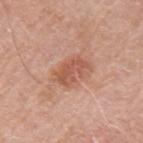Captured during whole-body skin photography for melanoma surveillance; the lesion was not biopsied. The lesion is located on the left upper arm. A lesion tile, about 15 mm wide, cut from a 3D total-body photograph. This is a white-light tile. A male patient, aged 78 to 82.